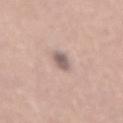Impression:
Part of a total-body skin-imaging series; this lesion was reviewed on a skin check and was not flagged for biopsy.
Background:
This is a white-light tile. The lesion is located on the back. The lesion's longest dimension is about 3 mm. The subject is a female approximately 50 years of age. Cropped from a total-body skin-imaging series; the visible field is about 15 mm. An algorithmic analysis of the crop reported a lesion area of about 4.5 mm² and a shape-asymmetry score of about 0.25 (0 = symmetric). And it measured a peripheral color-asymmetry measure near 1. It also reported an automated nevus-likeness rating near 5 out of 100 and a lesion-detection confidence of about 100/100.